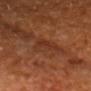No biopsy was performed on this lesion — it was imaged during a full skin examination and was not determined to be concerning.
A roughly 15 mm field-of-view crop from a total-body skin photograph.
The lesion-visualizer software estimated a mean CIELAB color near L≈33 a*≈24 b*≈30. And it measured a classifier nevus-likeness of about 0/100 and a detector confidence of about 70 out of 100 that the crop contains a lesion.
The lesion is located on the head or neck.
Captured under cross-polarized illumination.
A male patient, about 60 years old.